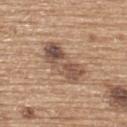– follow-up: imaged on a skin check; not biopsied
– TBP lesion metrics: a lesion color around L≈52 a*≈17 b*≈27 in CIELAB, a lesion–skin lightness drop of about 12, and a normalized border contrast of about 8.5; a border-irregularity rating of about 3.5/10, a color-variation rating of about 8.5/10, and peripheral color asymmetry of about 3
– anatomic site: the upper back
– acquisition: total-body-photography crop, ~15 mm field of view
– lesion size: ≈6 mm
– lighting: white-light illumination
– patient: male, approximately 65 years of age